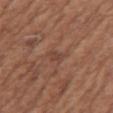notes: total-body-photography surveillance lesion; no biopsy
subject: female, aged 73 to 77
location: the left upper arm
illumination: white-light illumination
image: 15 mm crop, total-body photography
lesion diameter: ~2.5 mm (longest diameter)